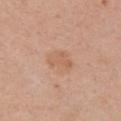| field | value |
|---|---|
| biopsy status | total-body-photography surveillance lesion; no biopsy |
| imaging modality | total-body-photography crop, ~15 mm field of view |
| location | the chest |
| subject | female, aged around 55 |
| tile lighting | white-light illumination |
| image-analysis metrics | a lesion–skin lightness drop of about 6 and a lesion-to-skin contrast of about 5 (normalized; higher = more distinct); a classifier nevus-likeness of about 0/100 and a detector confidence of about 100 out of 100 that the crop contains a lesion |